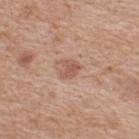Impression:
Recorded during total-body skin imaging; not selected for excision or biopsy.
Background:
A close-up tile cropped from a whole-body skin photograph, about 15 mm across. Approximately 2.5 mm at its widest. Automated image analysis of the tile measured a color-variation rating of about 2/10 and a peripheral color-asymmetry measure near 1. It also reported a nevus-likeness score of about 60/100. A female subject, aged 38–42. The lesion is located on the back. Imaged with white-light lighting.